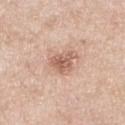notes: no biopsy performed (imaged during a skin exam)
site: the right lower leg
subject: female, aged 53–57
automated lesion analysis: an area of roughly 6 mm², an eccentricity of roughly 0.35, and a symmetry-axis asymmetry near 0.3; a lesion color around L≈60 a*≈21 b*≈28 in CIELAB, roughly 12 lightness units darker than nearby skin, and a lesion-to-skin contrast of about 7.5 (normalized; higher = more distinct); a border-irregularity index near 3/10, internal color variation of about 3 on a 0–10 scale, and a peripheral color-asymmetry measure near 1
imaging modality: 15 mm crop, total-body photography
size: ≈3 mm
illumination: white-light illumination